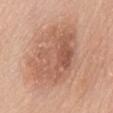<tbp_lesion>
<biopsy_status>not biopsied; imaged during a skin examination</biopsy_status>
<site>abdomen</site>
<lesion_size>
  <long_diameter_mm_approx>8.5</long_diameter_mm_approx>
</lesion_size>
<lighting>white-light</lighting>
<patient>
  <sex>female</sex>
  <age_approx>60</age_approx>
</patient>
<image>
  <source>total-body photography crop</source>
  <field_of_view_mm>15</field_of_view_mm>
</image>
</tbp_lesion>